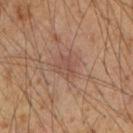<record>
<biopsy_status>not biopsied; imaged during a skin examination</biopsy_status>
<site>right upper arm</site>
<image>
  <source>total-body photography crop</source>
  <field_of_view_mm>15</field_of_view_mm>
</image>
<patient>
  <sex>male</sex>
  <age_approx>50</age_approx>
</patient>
</record>